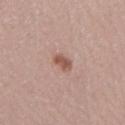Findings:
- follow-up — total-body-photography surveillance lesion; no biopsy
- imaging modality — total-body-photography crop, ~15 mm field of view
- diameter — about 2.5 mm
- patient — female, aged 38–42
- anatomic site — the right lower leg
- automated metrics — an average lesion color of about L≈54 a*≈21 b*≈26 (CIELAB) and a normalized lesion–skin contrast near 8